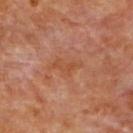From the upper back. The subject is a female approximately 50 years of age. A region of skin cropped from a whole-body photographic capture, roughly 15 mm wide. Captured under cross-polarized illumination. The lesion-visualizer software estimated a mean CIELAB color near L≈48 a*≈25 b*≈35, roughly 5 lightness units darker than nearby skin, and a normalized border contrast of about 5.5. The software also gave border irregularity of about 5.5 on a 0–10 scale and a within-lesion color-variation index near 1.5/10. The analysis additionally found an automated nevus-likeness rating near 0 out of 100 and a detector confidence of about 100 out of 100 that the crop contains a lesion.